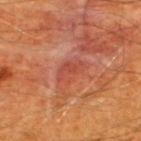Clinical impression: Imaged during a routine full-body skin examination; the lesion was not biopsied and no histopathology is available. Image and clinical context: Located on the upper back. A male patient aged 58 to 62. The recorded lesion diameter is about 3.5 mm. This image is a 15 mm lesion crop taken from a total-body photograph. Automated image analysis of the tile measured a detector confidence of about 95 out of 100 that the crop contains a lesion.